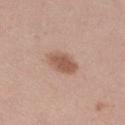Assessment: The lesion was tiled from a total-body skin photograph and was not biopsied. Context: This image is a 15 mm lesion crop taken from a total-body photograph. From the left lower leg. Approximately 4.5 mm at its widest. The tile uses white-light illumination. A female patient aged 23 to 27.